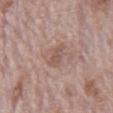Imaged during a routine full-body skin examination; the lesion was not biopsied and no histopathology is available.
On the abdomen.
A male subject, aged 68 to 72.
A 15 mm crop from a total-body photograph taken for skin-cancer surveillance.